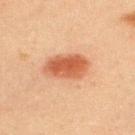Imaged during a routine full-body skin examination; the lesion was not biopsied and no histopathology is available.
Cropped from a whole-body photographic skin survey; the tile spans about 15 mm.
Imaged with cross-polarized lighting.
The lesion-visualizer software estimated a lesion area of about 13 mm². And it measured an average lesion color of about L≈49 a*≈25 b*≈33 (CIELAB). The software also gave a color-variation rating of about 4/10 and a peripheral color-asymmetry measure near 1. And it measured an automated nevus-likeness rating near 100 out of 100 and lesion-presence confidence of about 100/100.
The patient is a male aged 58 to 62.
The lesion is located on the upper back.
Approximately 5 mm at its widest.